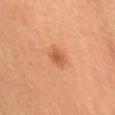No biopsy was performed on this lesion — it was imaged during a full skin examination and was not determined to be concerning.
The lesion is on the head or neck.
A female subject, aged approximately 40.
Imaged with cross-polarized lighting.
A 15 mm crop from a total-body photograph taken for skin-cancer surveillance.